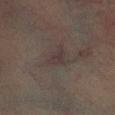Clinical impression: The lesion was tiled from a total-body skin photograph and was not biopsied. Acquisition and patient details: Located on the left lower leg. A roughly 15 mm field-of-view crop from a total-body skin photograph. Captured under cross-polarized illumination. The recorded lesion diameter is about 2.5 mm. A male patient about 40 years old.